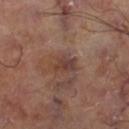workup: no biopsy performed (imaged during a skin exam); acquisition: total-body-photography crop, ~15 mm field of view; illumination: cross-polarized; location: the right thigh.